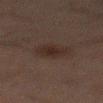Recorded during total-body skin imaging; not selected for excision or biopsy. A roughly 15 mm field-of-view crop from a total-body skin photograph. On the mid back. A male patient about 50 years old. An algorithmic analysis of the crop reported an area of roughly 5.5 mm², an eccentricity of roughly 0.25, and a shape-asymmetry score of about 0.25 (0 = symmetric). The analysis additionally found a classifier nevus-likeness of about 45/100 and lesion-presence confidence of about 100/100. Captured under cross-polarized illumination.